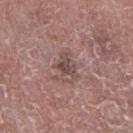illumination: white-light illumination | image-analysis metrics: a lesion area of about 4 mm², an outline eccentricity of about 0.75 (0 = round, 1 = elongated), and two-axis asymmetry of about 0.5; an automated nevus-likeness rating near 0 out of 100 and a lesion-detection confidence of about 100/100 | subject: male, about 75 years old | site: the right lower leg | image: ~15 mm crop, total-body skin-cancer survey | lesion diameter: ≈3 mm.